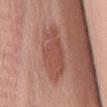Impression:
Captured during whole-body skin photography for melanoma surveillance; the lesion was not biopsied.
Clinical summary:
An algorithmic analysis of the crop reported internal color variation of about 3 on a 0–10 scale and peripheral color asymmetry of about 1. It also reported a lesion-detection confidence of about 100/100. On the chest. Cropped from a total-body skin-imaging series; the visible field is about 15 mm. The tile uses white-light illumination. About 7.5 mm across. The patient is a female aged around 60.